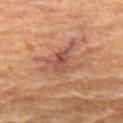Impression:
No biopsy was performed on this lesion — it was imaged during a full skin examination and was not determined to be concerning.
Background:
From the left thigh. A 15 mm close-up tile from a total-body photography series done for melanoma screening. A male patient approximately 60 years of age.